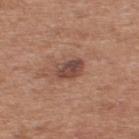workup: total-body-photography surveillance lesion; no biopsy | lesion size: about 3.5 mm | body site: the upper back | imaging modality: ~15 mm crop, total-body skin-cancer survey | patient: male, approximately 55 years of age.